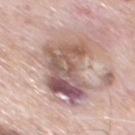| feature | finding |
|---|---|
| biopsy status | total-body-photography surveillance lesion; no biopsy |
| location | the front of the torso |
| subject | male, in their 80s |
| lesion diameter | ~9 mm (longest diameter) |
| acquisition | 15 mm crop, total-body photography |